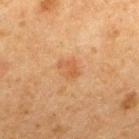Part of a total-body skin-imaging series; this lesion was reviewed on a skin check and was not flagged for biopsy.
The lesion is located on the upper back.
The tile uses cross-polarized illumination.
A male patient, aged 48 to 52.
A lesion tile, about 15 mm wide, cut from a 3D total-body photograph.